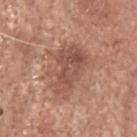biopsy_status: not biopsied; imaged during a skin examination
patient:
  sex: male
  age_approx: 80
image:
  source: total-body photography crop
  field_of_view_mm: 15
site: head or neck